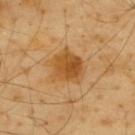biopsy status: total-body-photography surveillance lesion; no biopsy
lesion size: ≈4 mm
site: the upper back
subject: male, aged approximately 65
image: ~15 mm tile from a whole-body skin photo
tile lighting: cross-polarized